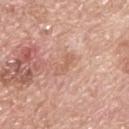{"biopsy_status": "not biopsied; imaged during a skin examination", "image": {"source": "total-body photography crop", "field_of_view_mm": 15}, "patient": {"sex": "male", "age_approx": 70}, "site": "upper back"}